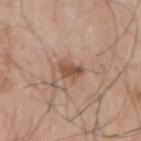follow-up: catalogued during a skin exam; not biopsied | subject: male, about 75 years old | lesion size: about 3.5 mm | anatomic site: the right upper arm | automated metrics: a footprint of about 5 mm² and an outline eccentricity of about 0.8 (0 = round, 1 = elongated); an average lesion color of about L≈52 a*≈19 b*≈29 (CIELAB) and a lesion–skin lightness drop of about 11; a classifier nevus-likeness of about 70/100 and lesion-presence confidence of about 100/100 | image: ~15 mm tile from a whole-body skin photo | tile lighting: white-light.